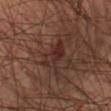Notes:
* workup — imaged on a skin check; not biopsied
* image — total-body-photography crop, ~15 mm field of view
* image-analysis metrics — a footprint of about 6.5 mm² and an eccentricity of roughly 0.7
* illumination — cross-polarized illumination
* body site — the left lower leg
* patient — male, in their 50s